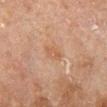Findings:
• biopsy status · catalogued during a skin exam; not biopsied
• illumination · cross-polarized
• location · the right lower leg
• lesion diameter · ≈2.5 mm
• image-analysis metrics · an eccentricity of roughly 0.85 and a shape-asymmetry score of about 0.25 (0 = symmetric); a mean CIELAB color near L≈47 a*≈18 b*≈29, a lesion–skin lightness drop of about 5, and a lesion-to-skin contrast of about 5 (normalized; higher = more distinct)
• patient · male, in their 70s
• image source · 15 mm crop, total-body photography